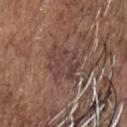lighting: white-light illumination; image: total-body-photography crop, ~15 mm field of view; subject: male, aged 73–77; anatomic site: the head or neck; image-analysis metrics: about 7 CIELAB-L* units darker than the surrounding skin and a normalized border contrast of about 6.5; size: about 4 mm.